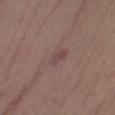biopsy status=total-body-photography surveillance lesion; no biopsy
body site=the right lower leg
TBP lesion metrics=a border-irregularity rating of about 2.5/10 and internal color variation of about 3 on a 0–10 scale; a classifier nevus-likeness of about 0/100 and a lesion-detection confidence of about 100/100
illumination=white-light
diameter=~3 mm (longest diameter)
patient=male, aged 73 to 77
image source=~15 mm crop, total-body skin-cancer survey